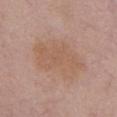workup=no biopsy performed (imaged during a skin exam)
tile lighting=white-light illumination
site=the back
lesion diameter=≈7 mm
patient=female, aged approximately 65
acquisition=~15 mm tile from a whole-body skin photo
image-analysis metrics=a shape eccentricity near 0.7 and a symmetry-axis asymmetry near 0.25; internal color variation of about 2.5 on a 0–10 scale and a peripheral color-asymmetry measure near 1; a classifier nevus-likeness of about 0/100 and lesion-presence confidence of about 100/100